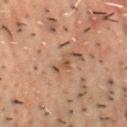biopsy status: catalogued during a skin exam; not biopsied
illumination: cross-polarized illumination
diameter: ≈2.5 mm
anatomic site: the chest
subject: male, aged approximately 50
image source: ~15 mm tile from a whole-body skin photo